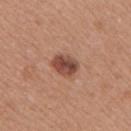Part of a total-body skin-imaging series; this lesion was reviewed on a skin check and was not flagged for biopsy.
A female patient approximately 35 years of age.
Imaged with white-light lighting.
On the right upper arm.
A lesion tile, about 15 mm wide, cut from a 3D total-body photograph.